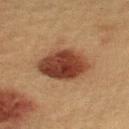<case>
<biopsy_status>not biopsied; imaged during a skin examination</biopsy_status>
</case>